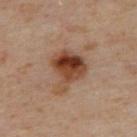Cropped from a total-body skin-imaging series; the visible field is about 15 mm.
A female subject, about 60 years old.
The lesion is on the upper back.
About 5 mm across.
Imaged with cross-polarized lighting.
The total-body-photography lesion software estimated an area of roughly 14 mm², an outline eccentricity of about 0.6 (0 = round, 1 = elongated), and a shape-asymmetry score of about 0.45 (0 = symmetric). And it measured a mean CIELAB color near L≈43 a*≈21 b*≈31 and a lesion–skin lightness drop of about 14. The analysis additionally found border irregularity of about 4.5 on a 0–10 scale and a color-variation rating of about 9/10.